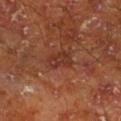Assessment:
This lesion was catalogued during total-body skin photography and was not selected for biopsy.
Image and clinical context:
A roughly 15 mm field-of-view crop from a total-body skin photograph. The total-body-photography lesion software estimated an area of roughly 5.5 mm², a shape eccentricity near 0.8, and a shape-asymmetry score of about 0.15 (0 = symmetric). It also reported a lesion color around L≈33 a*≈25 b*≈27 in CIELAB, roughly 7 lightness units darker than nearby skin, and a normalized lesion–skin contrast near 6.5. And it measured a border-irregularity index near 2/10, a within-lesion color-variation index near 3.5/10, and radial color variation of about 1. The software also gave an automated nevus-likeness rating near 0 out of 100 and a detector confidence of about 100 out of 100 that the crop contains a lesion. A male patient, aged 63 to 67. This is a cross-polarized tile. The lesion is on the leg. Longest diameter approximately 3 mm.